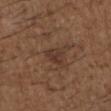workup: imaged on a skin check; not biopsied
lesion diameter: about 2.5 mm
subject: male, roughly 50 years of age
image: 15 mm crop, total-body photography
site: the arm